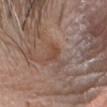The lesion was photographed on a routine skin check and not biopsied; there is no pathology result. The lesion's longest dimension is about 2.5 mm. The lesion is located on the head or neck. A lesion tile, about 15 mm wide, cut from a 3D total-body photograph. This is a white-light tile. The patient is a male in their 80s.